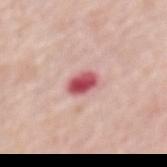Q: Was this lesion biopsied?
A: no biopsy performed (imaged during a skin exam)
Q: Where on the body is the lesion?
A: the mid back
Q: What lighting was used for the tile?
A: white-light
Q: Lesion size?
A: ≈3 mm
Q: What are the patient's age and sex?
A: female, aged 63–67
Q: What kind of image is this?
A: ~15 mm crop, total-body skin-cancer survey